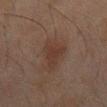Impression: No biopsy was performed on this lesion — it was imaged during a full skin examination and was not determined to be concerning. Image and clinical context: A male subject, aged 43 to 47. A 15 mm crop from a total-body photograph taken for skin-cancer surveillance. The total-body-photography lesion software estimated a nevus-likeness score of about 30/100 and a lesion-detection confidence of about 100/100. Located on the back. Approximately 4.5 mm at its widest. Imaged with cross-polarized lighting.